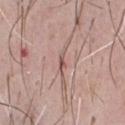Q: Is there a histopathology result?
A: imaged on a skin check; not biopsied
Q: How was this image acquired?
A: 15 mm crop, total-body photography
Q: Patient demographics?
A: male, roughly 50 years of age
Q: Lesion size?
A: ≈3.5 mm
Q: How was the tile lit?
A: white-light
Q: Lesion location?
A: the chest